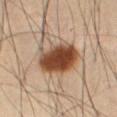Clinical summary:
A roughly 15 mm field-of-view crop from a total-body skin photograph. A male subject in their mid- to late 50s. The tile uses cross-polarized illumination. The lesion's longest dimension is about 5.5 mm. On the abdomen.